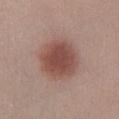This lesion was catalogued during total-body skin photography and was not selected for biopsy. A 15 mm crop from a total-body photograph taken for skin-cancer surveillance. Automated image analysis of the tile measured an area of roughly 20 mm², an eccentricity of roughly 0.4, and a symmetry-axis asymmetry near 0.1. The software also gave a lesion color around L≈48 a*≈21 b*≈24 in CIELAB, a lesion–skin lightness drop of about 12, and a normalized lesion–skin contrast near 8.5. The analysis additionally found border irregularity of about 1 on a 0–10 scale, a within-lesion color-variation index near 3.5/10, and peripheral color asymmetry of about 1. The analysis additionally found a nevus-likeness score of about 100/100 and lesion-presence confidence of about 100/100. A female subject aged 23–27. About 5 mm across. The lesion is on the right lower leg.